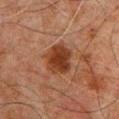No biopsy was performed on this lesion — it was imaged during a full skin examination and was not determined to be concerning.
From the front of the torso.
A lesion tile, about 15 mm wide, cut from a 3D total-body photograph.
The lesion's longest dimension is about 4 mm.
Captured under cross-polarized illumination.
A male patient, aged around 60.
Automated tile analysis of the lesion measured a lesion area of about 10 mm², a shape eccentricity near 0.55, and a shape-asymmetry score of about 0.2 (0 = symmetric). The analysis additionally found a mean CIELAB color near L≈29 a*≈20 b*≈27, roughly 10 lightness units darker than nearby skin, and a lesion-to-skin contrast of about 10 (normalized; higher = more distinct).